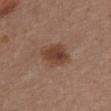Assessment:
This lesion was catalogued during total-body skin photography and was not selected for biopsy.
Context:
Approximately 3.5 mm at its widest. Imaged with white-light lighting. A 15 mm close-up extracted from a 3D total-body photography capture. Located on the chest. A female patient, roughly 30 years of age.